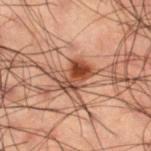  biopsy_status: not biopsied; imaged during a skin examination
  lighting: cross-polarized
  image:
    source: total-body photography crop
    field_of_view_mm: 15
  site: leg
  patient:
    sex: male
    age_approx: 50
  automated_metrics:
    area_mm2_approx: 7.5
    eccentricity: 0.7
    shape_asymmetry: 0.3
  lesion_size:
    long_diameter_mm_approx: 3.5